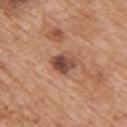Impression: Recorded during total-body skin imaging; not selected for excision or biopsy. Image and clinical context: A male subject in their 60s. A roughly 15 mm field-of-view crop from a total-body skin photograph. The lesion's longest dimension is about 3 mm. The lesion-visualizer software estimated a lesion area of about 5.5 mm², an eccentricity of roughly 0.75, and a shape-asymmetry score of about 0.3 (0 = symmetric). It also reported a mean CIELAB color near L≈47 a*≈23 b*≈28, a lesion–skin lightness drop of about 14, and a normalized border contrast of about 10.5. The analysis additionally found a peripheral color-asymmetry measure near 2.5. It also reported a lesion-detection confidence of about 100/100. This is a white-light tile. The lesion is on the upper back.